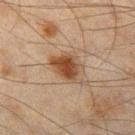workup=catalogued during a skin exam; not biopsied | patient=male, about 45 years old | lighting=cross-polarized | image source=total-body-photography crop, ~15 mm field of view | body site=the left thigh.